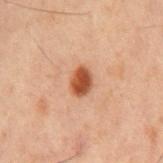Clinical impression: Recorded during total-body skin imaging; not selected for excision or biopsy. Clinical summary: This is a cross-polarized tile. A lesion tile, about 15 mm wide, cut from a 3D total-body photograph. The patient is a male aged 58 to 62. Measured at roughly 3 mm in maximum diameter. The lesion is on the mid back. Automated image analysis of the tile measured roughly 13 lightness units darker than nearby skin and a normalized lesion–skin contrast near 11. It also reported border irregularity of about 2 on a 0–10 scale and a within-lesion color-variation index near 3/10. It also reported a nevus-likeness score of about 100/100 and lesion-presence confidence of about 100/100.